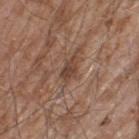biopsy status: imaged on a skin check; not biopsied
tile lighting: white-light
subject: male, roughly 60 years of age
image-analysis metrics: an area of roughly 4.5 mm², a shape eccentricity near 0.85, and a shape-asymmetry score of about 0.25 (0 = symmetric); a lesion-to-skin contrast of about 7.5 (normalized; higher = more distinct); a border-irregularity rating of about 3/10 and peripheral color asymmetry of about 1
diameter: ~3 mm (longest diameter)
acquisition: total-body-photography crop, ~15 mm field of view
site: the leg